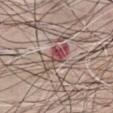Clinical impression:
Imaged during a routine full-body skin examination; the lesion was not biopsied and no histopathology is available.
Context:
The recorded lesion diameter is about 5 mm. The tile uses white-light illumination. The total-body-photography lesion software estimated an average lesion color of about L≈50 a*≈22 b*≈21 (CIELAB), roughly 11 lightness units darker than nearby skin, and a lesion-to-skin contrast of about 8 (normalized; higher = more distinct). The analysis additionally found border irregularity of about 4.5 on a 0–10 scale, a color-variation rating of about 10/10, and a peripheral color-asymmetry measure near 4.5. It also reported an automated nevus-likeness rating near 0 out of 100 and lesion-presence confidence of about 100/100. The patient is a male approximately 70 years of age. This image is a 15 mm lesion crop taken from a total-body photograph. The lesion is on the chest.